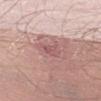Captured during whole-body skin photography for melanoma surveillance; the lesion was not biopsied. The lesion is on the leg. A male subject approximately 50 years of age. A roughly 15 mm field-of-view crop from a total-body skin photograph.